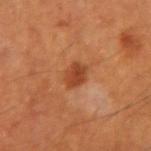The lesion is located on the right upper arm.
The lesion's longest dimension is about 3.5 mm.
This is a cross-polarized tile.
A region of skin cropped from a whole-body photographic capture, roughly 15 mm wide.
The patient is a male roughly 60 years of age.